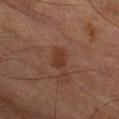Assessment: Part of a total-body skin-imaging series; this lesion was reviewed on a skin check and was not flagged for biopsy. Context: Imaged with cross-polarized lighting. The subject is a male about 85 years old or older. Cropped from a whole-body photographic skin survey; the tile spans about 15 mm. Located on the right lower leg. The total-body-photography lesion software estimated a border-irregularity index near 2/10 and a peripheral color-asymmetry measure near 0.5. It also reported an automated nevus-likeness rating near 15 out of 100 and a lesion-detection confidence of about 100/100. About 2.5 mm across.